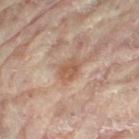Part of a total-body skin-imaging series; this lesion was reviewed on a skin check and was not flagged for biopsy. Measured at roughly 3 mm in maximum diameter. This is a cross-polarized tile. A lesion tile, about 15 mm wide, cut from a 3D total-body photograph. From the right leg. A female patient, approximately 80 years of age. Automated image analysis of the tile measured an average lesion color of about L≈50 a*≈18 b*≈27 (CIELAB), about 8 CIELAB-L* units darker than the surrounding skin, and a lesion-to-skin contrast of about 6.5 (normalized; higher = more distinct). And it measured a nevus-likeness score of about 0/100 and a detector confidence of about 100 out of 100 that the crop contains a lesion.